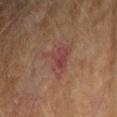No biopsy was performed on this lesion — it was imaged during a full skin examination and was not determined to be concerning. The tile uses cross-polarized illumination. The lesion's longest dimension is about 4.5 mm. A female patient, aged around 60. Cropped from a total-body skin-imaging series; the visible field is about 15 mm. On the left forearm.